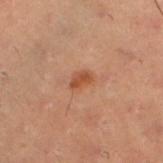Notes:
- follow-up — total-body-photography surveillance lesion; no biopsy
- acquisition — ~15 mm crop, total-body skin-cancer survey
- anatomic site — the left lower leg
- subject — male, roughly 30 years of age